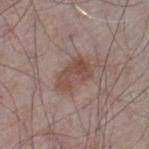No biopsy was performed on this lesion — it was imaged during a full skin examination and was not determined to be concerning. The total-body-photography lesion software estimated a footprint of about 9.5 mm², an outline eccentricity of about 0.8 (0 = round, 1 = elongated), and a shape-asymmetry score of about 0.25 (0 = symmetric). And it measured a mean CIELAB color near L≈48 a*≈18 b*≈23, about 8 CIELAB-L* units darker than the surrounding skin, and a normalized border contrast of about 7. It also reported a border-irregularity rating of about 3/10, a within-lesion color-variation index near 3.5/10, and peripheral color asymmetry of about 1.5. The tile uses white-light illumination. Located on the left thigh. The subject is a male about 65 years old. A 15 mm close-up tile from a total-body photography series done for melanoma screening. The lesion's longest dimension is about 4.5 mm.